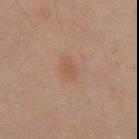No biopsy was performed on this lesion — it was imaged during a full skin examination and was not determined to be concerning.
A male subject, approximately 45 years of age.
A region of skin cropped from a whole-body photographic capture, roughly 15 mm wide.
The recorded lesion diameter is about 3 mm.
Automated tile analysis of the lesion measured a mean CIELAB color near L≈42 a*≈16 b*≈25, a lesion–skin lightness drop of about 5, and a normalized lesion–skin contrast near 5. And it measured a border-irregularity index near 1.5/10, a color-variation rating of about 1.5/10, and radial color variation of about 0.5.
From the mid back.
Imaged with cross-polarized lighting.